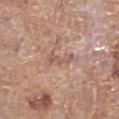Captured during whole-body skin photography for melanoma surveillance; the lesion was not biopsied.
Cropped from a total-body skin-imaging series; the visible field is about 15 mm.
Measured at roughly 4 mm in maximum diameter.
Located on the left lower leg.
An algorithmic analysis of the crop reported an average lesion color of about L≈57 a*≈20 b*≈25 (CIELAB), about 7 CIELAB-L* units darker than the surrounding skin, and a normalized lesion–skin contrast near 5. And it measured a border-irregularity rating of about 5/10, a within-lesion color-variation index near 2/10, and peripheral color asymmetry of about 0.5.
The tile uses white-light illumination.
A female subject aged 73 to 77.